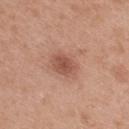biopsy status — catalogued during a skin exam; not biopsied | diameter — ≈4.5 mm | subject — female, aged 38 to 42 | lighting — white-light | image source — total-body-photography crop, ~15 mm field of view | automated metrics — a lesion color around L≈56 a*≈22 b*≈28 in CIELAB, a lesion–skin lightness drop of about 9, and a lesion-to-skin contrast of about 6 (normalized; higher = more distinct); a detector confidence of about 100 out of 100 that the crop contains a lesion | location — the upper back.